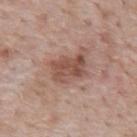Q: Was a biopsy performed?
A: imaged on a skin check; not biopsied
Q: Patient demographics?
A: male, in their mid-50s
Q: Lesion size?
A: ~4 mm (longest diameter)
Q: What kind of image is this?
A: ~15 mm tile from a whole-body skin photo
Q: Where on the body is the lesion?
A: the back
Q: What lighting was used for the tile?
A: white-light illumination